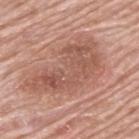<record>
  <lesion_size>
    <long_diameter_mm_approx>9.5</long_diameter_mm_approx>
  </lesion_size>
  <site>upper back</site>
  <image>
    <source>total-body photography crop</source>
    <field_of_view_mm>15</field_of_view_mm>
  </image>
  <patient>
    <sex>male</sex>
    <age_approx>80</age_approx>
  </patient>
</record>